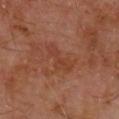workup: imaged on a skin check; not biopsied
tile lighting: cross-polarized
image source: 15 mm crop, total-body photography
lesion diameter: ~4 mm (longest diameter)
location: the upper back
subject: male, in their 70s